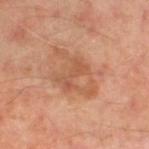Clinical impression: Recorded during total-body skin imaging; not selected for excision or biopsy. Acquisition and patient details: Automated tile analysis of the lesion measured a mean CIELAB color near L≈55 a*≈24 b*≈34 and a normalized border contrast of about 5.5. From the left leg. Imaged with cross-polarized lighting. A male subject approximately 50 years of age. This image is a 15 mm lesion crop taken from a total-body photograph. The lesion's longest dimension is about 6.5 mm.